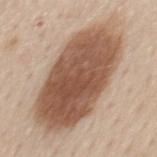Assessment: This lesion was catalogued during total-body skin photography and was not selected for biopsy. Image and clinical context: The patient is a male aged 58–62. On the mid back. This image is a 15 mm lesion crop taken from a total-body photograph.